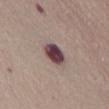Q: Was a biopsy performed?
A: catalogued during a skin exam; not biopsied